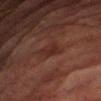Part of a total-body skin-imaging series; this lesion was reviewed on a skin check and was not flagged for biopsy.
The subject is a male about 85 years old or older.
Cropped from a total-body skin-imaging series; the visible field is about 15 mm.
The recorded lesion diameter is about 3 mm.
The tile uses cross-polarized illumination.
On the right thigh.